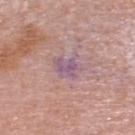Recorded during total-body skin imaging; not selected for excision or biopsy. About 3 mm across. The patient is a female approximately 60 years of age. The lesion is on the head or neck. A 15 mm crop from a total-body photograph taken for skin-cancer surveillance.